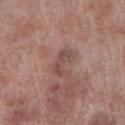{"biopsy_status": "not biopsied; imaged during a skin examination", "site": "right lower leg", "patient": {"sex": "male", "age_approx": 70}, "lesion_size": {"long_diameter_mm_approx": 3.5}, "image": {"source": "total-body photography crop", "field_of_view_mm": 15}}